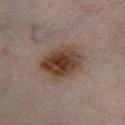Imaged during a routine full-body skin examination; the lesion was not biopsied and no histopathology is available.